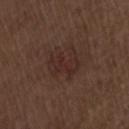Recorded during total-body skin imaging; not selected for excision or biopsy. A male patient, in their 70s. Captured under white-light illumination. Automated image analysis of the tile measured a detector confidence of about 100 out of 100 that the crop contains a lesion. Located on the lower back. A lesion tile, about 15 mm wide, cut from a 3D total-body photograph. The recorded lesion diameter is about 3.5 mm.